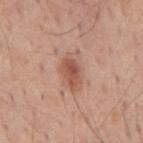Q: Was a biopsy performed?
A: imaged on a skin check; not biopsied
Q: Automated lesion metrics?
A: a border-irregularity rating of about 3.5/10, internal color variation of about 4 on a 0–10 scale, and a peripheral color-asymmetry measure near 1; a nevus-likeness score of about 90/100 and lesion-presence confidence of about 100/100
Q: Where on the body is the lesion?
A: the mid back
Q: What is the imaging modality?
A: ~15 mm crop, total-body skin-cancer survey
Q: What are the patient's age and sex?
A: male, aged 58–62
Q: Illumination type?
A: white-light